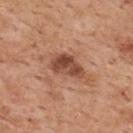Impression: Captured during whole-body skin photography for melanoma surveillance; the lesion was not biopsied. Image and clinical context: A 15 mm crop from a total-body photograph taken for skin-cancer surveillance. A male patient, roughly 60 years of age. The tile uses white-light illumination. The lesion's longest dimension is about 4.5 mm. From the upper back.